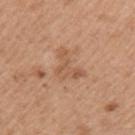Assessment:
No biopsy was performed on this lesion — it was imaged during a full skin examination and was not determined to be concerning.
Context:
This is a white-light tile. A female subject, aged 38 to 42. About 4 mm across. From the left upper arm. A 15 mm crop from a total-body photograph taken for skin-cancer surveillance.